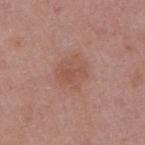follow-up = no biopsy performed (imaged during a skin exam)
patient = female, aged approximately 40
anatomic site = the arm
imaging modality = total-body-photography crop, ~15 mm field of view